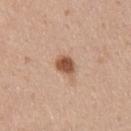Recorded during total-body skin imaging; not selected for excision or biopsy.
A male subject, aged 33–37.
Approximately 3 mm at its widest.
A 15 mm close-up extracted from a 3D total-body photography capture.
Automated tile analysis of the lesion measured a footprint of about 5 mm² and an eccentricity of roughly 0.7. The software also gave a lesion color around L≈54 a*≈22 b*≈32 in CIELAB and a lesion–skin lightness drop of about 15.
This is a white-light tile.
From the right upper arm.